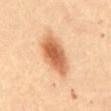Notes:
– subject: female, aged around 50
– image: total-body-photography crop, ~15 mm field of view
– diameter: ≈6 mm
– body site: the abdomen